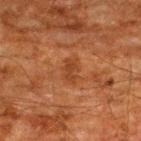Impression:
Captured during whole-body skin photography for melanoma surveillance; the lesion was not biopsied.
Background:
Imaged with cross-polarized lighting. This image is a 15 mm lesion crop taken from a total-body photograph. An algorithmic analysis of the crop reported an outline eccentricity of about 0.85 (0 = round, 1 = elongated). And it measured a mean CIELAB color near L≈32 a*≈22 b*≈31 and a lesion-to-skin contrast of about 6 (normalized; higher = more distinct). And it measured a border-irregularity rating of about 3.5/10. The software also gave a nevus-likeness score of about 0/100 and a detector confidence of about 100 out of 100 that the crop contains a lesion. The lesion's longest dimension is about 2.5 mm. A male patient aged 58–62. The lesion is on the back.